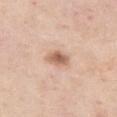Part of a total-body skin-imaging series; this lesion was reviewed on a skin check and was not flagged for biopsy. Longest diameter approximately 3 mm. Imaged with white-light lighting. A lesion tile, about 15 mm wide, cut from a 3D total-body photograph. Located on the leg. A female subject, aged around 55.